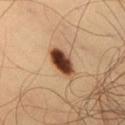{
  "biopsy_status": "not biopsied; imaged during a skin examination",
  "image": {
    "source": "total-body photography crop",
    "field_of_view_mm": 15
  },
  "patient": {
    "sex": "male",
    "age_approx": 35
  },
  "lighting": "cross-polarized",
  "site": "chest",
  "automated_metrics": {
    "vs_skin_darker_L": 22.0,
    "vs_skin_contrast_norm": 15.0,
    "nevus_likeness_0_100": 100,
    "lesion_detection_confidence_0_100": 100
  }
}